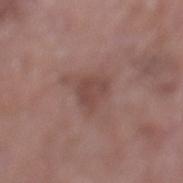Captured during whole-body skin photography for melanoma surveillance; the lesion was not biopsied. Cropped from a total-body skin-imaging series; the visible field is about 15 mm. The patient is a female aged 83 or over. From the left lower leg.